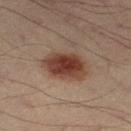diameter — about 5 mm | image-analysis metrics — a lesion area of about 14 mm², an eccentricity of roughly 0.75, and a symmetry-axis asymmetry near 0.2; a border-irregularity rating of about 2/10, a within-lesion color-variation index near 4.5/10, and radial color variation of about 1.5; a nevus-likeness score of about 100/100 | illumination — cross-polarized illumination | acquisition — 15 mm crop, total-body photography | site — the left thigh | patient — male, aged 38–42.